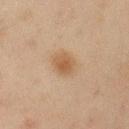A close-up tile cropped from a whole-body skin photograph, about 15 mm across. The recorded lesion diameter is about 2.5 mm. The lesion is on the left upper arm. The patient is a male in their mid- to late 40s. Imaged with cross-polarized lighting. Automated image analysis of the tile measured a footprint of about 5 mm², an outline eccentricity of about 0.45 (0 = round, 1 = elongated), and two-axis asymmetry of about 0.2. It also reported a border-irregularity rating of about 2/10, a within-lesion color-variation index near 2/10, and radial color variation of about 0.5. The software also gave a detector confidence of about 100 out of 100 that the crop contains a lesion.